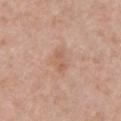Case summary:
* notes — total-body-photography surveillance lesion; no biopsy
* size — about 3 mm
* lighting — white-light illumination
* patient — female, about 40 years old
* image source — ~15 mm tile from a whole-body skin photo
* site — the chest